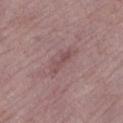Part of a total-body skin-imaging series; this lesion was reviewed on a skin check and was not flagged for biopsy. On the left lower leg. Cropped from a whole-body photographic skin survey; the tile spans about 15 mm. A female patient, aged 63–67. The total-body-photography lesion software estimated an average lesion color of about L≈49 a*≈21 b*≈18 (CIELAB), a lesion–skin lightness drop of about 7, and a lesion-to-skin contrast of about 5.5 (normalized; higher = more distinct).